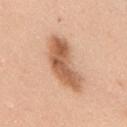Findings:
- biopsy status — catalogued during a skin exam; not biopsied
- lesion diameter — ~7.5 mm (longest diameter)
- acquisition — 15 mm crop, total-body photography
- illumination — white-light illumination
- body site — the upper back
- subject — male, aged around 35
- automated metrics — an average lesion color of about L≈61 a*≈22 b*≈34 (CIELAB), a lesion–skin lightness drop of about 14, and a normalized border contrast of about 9; border irregularity of about 4 on a 0–10 scale, internal color variation of about 6 on a 0–10 scale, and radial color variation of about 2.5; an automated nevus-likeness rating near 85 out of 100 and a detector confidence of about 100 out of 100 that the crop contains a lesion